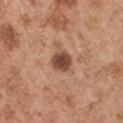Q: Was this lesion biopsied?
A: no biopsy performed (imaged during a skin exam)
Q: What lighting was used for the tile?
A: white-light illumination
Q: How was this image acquired?
A: 15 mm crop, total-body photography
Q: Who is the patient?
A: male, roughly 55 years of age
Q: What did automated image analysis measure?
A: a lesion color around L≈46 a*≈24 b*≈29 in CIELAB, roughly 15 lightness units darker than nearby skin, and a normalized lesion–skin contrast near 11; a border-irregularity rating of about 2/10, a color-variation rating of about 2.5/10, and peripheral color asymmetry of about 0.5
Q: Lesion location?
A: the left upper arm
Q: Lesion size?
A: ~2.5 mm (longest diameter)